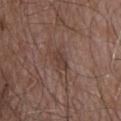follow-up: no biopsy performed (imaged during a skin exam) | site: the upper back | patient: male, aged 53–57 | tile lighting: white-light illumination | image-analysis metrics: a footprint of about 4 mm²; a border-irregularity rating of about 3/10 and a color-variation rating of about 1.5/10; a classifier nevus-likeness of about 0/100 and lesion-presence confidence of about 100/100 | lesion size: ≈2.5 mm | acquisition: 15 mm crop, total-body photography.